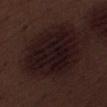Case summary:
• follow-up · imaged on a skin check; not biopsied
• site · the right thigh
• subject · male, aged around 70
• illumination · white-light illumination
• image · ~15 mm crop, total-body skin-cancer survey
• lesion size · about 9.5 mm